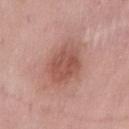follow-up: imaged on a skin check; not biopsied
lighting: white-light
image source: total-body-photography crop, ~15 mm field of view
automated lesion analysis: an area of roughly 15 mm², an outline eccentricity of about 0.65 (0 = round, 1 = elongated), and a symmetry-axis asymmetry near 0.15; a lesion–skin lightness drop of about 10; a nevus-likeness score of about 35/100 and a lesion-detection confidence of about 100/100
anatomic site: the mid back
subject: male, approximately 55 years of age